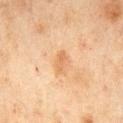This lesion was catalogued during total-body skin photography and was not selected for biopsy.
This image is a 15 mm lesion crop taken from a total-body photograph.
A male subject, aged 43 to 47.
An algorithmic analysis of the crop reported a footprint of about 2.5 mm², a shape eccentricity near 0.9, and two-axis asymmetry of about 0.35. And it measured about 7 CIELAB-L* units darker than the surrounding skin. And it measured an automated nevus-likeness rating near 5 out of 100.
Captured under cross-polarized illumination.
From the mid back.